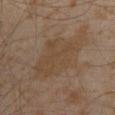Captured during whole-body skin photography for melanoma surveillance; the lesion was not biopsied.
This image is a 15 mm lesion crop taken from a total-body photograph.
The lesion is located on the front of the torso.
Approximately 8.5 mm at its widest.
An algorithmic analysis of the crop reported a footprint of about 25 mm², a shape eccentricity near 0.8, and a symmetry-axis asymmetry near 0.25. The analysis additionally found a lesion color around L≈39 a*≈13 b*≈26 in CIELAB, a lesion–skin lightness drop of about 5, and a lesion-to-skin contrast of about 5.5 (normalized; higher = more distinct). The analysis additionally found a border-irregularity rating of about 4/10 and a within-lesion color-variation index near 2/10. The software also gave a classifier nevus-likeness of about 0/100 and a detector confidence of about 100 out of 100 that the crop contains a lesion.
Captured under cross-polarized illumination.
The patient is a male in their 60s.